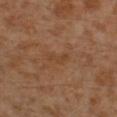Assessment: This lesion was catalogued during total-body skin photography and was not selected for biopsy. Background: This image is a 15 mm lesion crop taken from a total-body photograph. Approximately 2.5 mm at its widest. The subject is a male roughly 30 years of age. On the left leg. An algorithmic analysis of the crop reported a mean CIELAB color near L≈40 a*≈19 b*≈32 and a lesion-to-skin contrast of about 5 (normalized; higher = more distinct). And it measured border irregularity of about 5.5 on a 0–10 scale, internal color variation of about 0 on a 0–10 scale, and radial color variation of about 0. This is a cross-polarized tile.